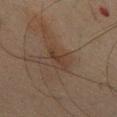Part of a total-body skin-imaging series; this lesion was reviewed on a skin check and was not flagged for biopsy.
A male patient, roughly 65 years of age.
The lesion is located on the chest.
A close-up tile cropped from a whole-body skin photograph, about 15 mm across.
Automated image analysis of the tile measured an outline eccentricity of about 0.85 (0 = round, 1 = elongated) and a symmetry-axis asymmetry near 0.3. And it measured an automated nevus-likeness rating near 0 out of 100 and lesion-presence confidence of about 100/100.